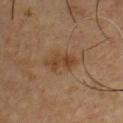Notes:
- follow-up: catalogued during a skin exam; not biopsied
- size: ≈4 mm
- image: 15 mm crop, total-body photography
- lighting: cross-polarized
- site: the front of the torso
- subject: male, approximately 55 years of age